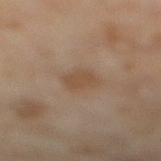Impression: No biopsy was performed on this lesion — it was imaged during a full skin examination and was not determined to be concerning. Background: A region of skin cropped from a whole-body photographic capture, roughly 15 mm wide. From the right lower leg. A female patient, approximately 60 years of age.